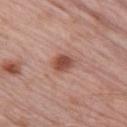Assessment:
The lesion was photographed on a routine skin check and not biopsied; there is no pathology result.
Acquisition and patient details:
The subject is a male in their mid- to late 60s. This is a white-light tile. Automated tile analysis of the lesion measured a lesion area of about 5 mm², an outline eccentricity of about 0.35 (0 = round, 1 = elongated), and a symmetry-axis asymmetry near 0.15. It also reported border irregularity of about 1.5 on a 0–10 scale, a color-variation rating of about 2.5/10, and radial color variation of about 0.5. The analysis additionally found a lesion-detection confidence of about 100/100. This image is a 15 mm lesion crop taken from a total-body photograph. The recorded lesion diameter is about 2.5 mm. The lesion is located on the arm.